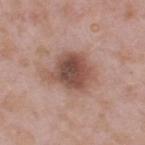Case summary:
• notes: catalogued during a skin exam; not biopsied
• lesion size: ≈5.5 mm
• anatomic site: the upper back
• imaging modality: 15 mm crop, total-body photography
• patient: male, aged approximately 55